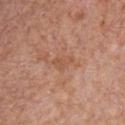| key | value |
|---|---|
| follow-up | total-body-photography surveillance lesion; no biopsy |
| automated lesion analysis | a footprint of about 3 mm², an outline eccentricity of about 0.85 (0 = round, 1 = elongated), and a shape-asymmetry score of about 0.5 (0 = symmetric); an average lesion color of about L≈53 a*≈23 b*≈32 (CIELAB) and a normalized lesion–skin contrast near 5; a border-irregularity rating of about 4.5/10, a color-variation rating of about 0.5/10, and a peripheral color-asymmetry measure near 0 |
| imaging modality | 15 mm crop, total-body photography |
| site | the chest |
| subject | male, in their mid-60s |